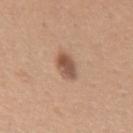| key | value |
|---|---|
| biopsy status | imaged on a skin check; not biopsied |
| location | the right upper arm |
| acquisition | total-body-photography crop, ~15 mm field of view |
| image-analysis metrics | border irregularity of about 2 on a 0–10 scale, a within-lesion color-variation index near 3.5/10, and peripheral color asymmetry of about 1; a nevus-likeness score of about 95/100 and a lesion-detection confidence of about 100/100 |
| subject | female, about 30 years old |
| lesion size | ~2.5 mm (longest diameter) |
| illumination | white-light |